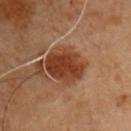Part of a total-body skin-imaging series; this lesion was reviewed on a skin check and was not flagged for biopsy. From the chest. A 15 mm close-up tile from a total-body photography series done for melanoma screening. Captured under cross-polarized illumination. A male patient roughly 50 years of age. Automated tile analysis of the lesion measured a footprint of about 15 mm² and an eccentricity of roughly 0.5. It also reported an average lesion color of about L≈40 a*≈25 b*≈33 (CIELAB), roughly 12 lightness units darker than nearby skin, and a normalized lesion–skin contrast near 10.